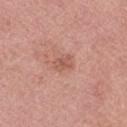workup: total-body-photography surveillance lesion; no biopsy
patient: female, aged approximately 70
image source: ~15 mm tile from a whole-body skin photo
TBP lesion metrics: a mean CIELAB color near L≈56 a*≈24 b*≈28, a lesion–skin lightness drop of about 8, and a normalized border contrast of about 5.5; a lesion-detection confidence of about 100/100
lesion size: ~2.5 mm (longest diameter)
location: the right thigh